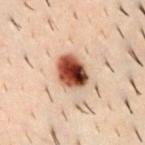The lesion was tiled from a total-body skin photograph and was not biopsied. The lesion is located on the chest. The patient is a male in their 30s. Captured under cross-polarized illumination. A close-up tile cropped from a whole-body skin photograph, about 15 mm across.